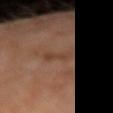A lesion tile, about 15 mm wide, cut from a 3D total-body photograph.
The subject is a female aged around 65.
The recorded lesion diameter is about 3.5 mm.
The tile uses cross-polarized illumination.
Located on the right forearm.